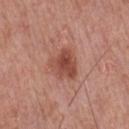biopsy status: total-body-photography surveillance lesion; no biopsy
body site: the chest
patient: male, aged approximately 70
size: ≈3.5 mm
automated metrics: a lesion area of about 9 mm² and a shape eccentricity near 0.5; a lesion–skin lightness drop of about 11 and a normalized border contrast of about 8; a border-irregularity rating of about 2.5/10 and a color-variation rating of about 4.5/10
image source: ~15 mm tile from a whole-body skin photo